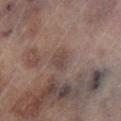Recorded during total-body skin imaging; not selected for excision or biopsy. This is a cross-polarized tile. Located on the left lower leg. A region of skin cropped from a whole-body photographic capture, roughly 15 mm wide. The total-body-photography lesion software estimated an average lesion color of about L≈41 a*≈14 b*≈19 (CIELAB), roughly 6 lightness units darker than nearby skin, and a normalized lesion–skin contrast near 5.5. The analysis additionally found border irregularity of about 2.5 on a 0–10 scale, a color-variation rating of about 1.5/10, and radial color variation of about 0.5. A male patient aged 63 to 67. The lesion's longest dimension is about 2.5 mm.